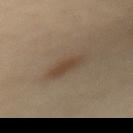• notes — catalogued during a skin exam; not biopsied
• image source — ~15 mm crop, total-body skin-cancer survey
• subject — female, aged 43–47
• tile lighting — cross-polarized
• automated lesion analysis — a mean CIELAB color near L≈44 a*≈14 b*≈28 and a normalized lesion–skin contrast near 7.5; border irregularity of about 4 on a 0–10 scale, a within-lesion color-variation index near 2/10, and a peripheral color-asymmetry measure near 0.5; an automated nevus-likeness rating near 90 out of 100 and a lesion-detection confidence of about 95/100
• site — the mid back